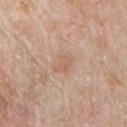anatomic site=the chest
imaging modality=~15 mm tile from a whole-body skin photo
patient=male, aged around 60